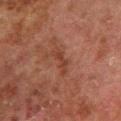{"biopsy_status": "not biopsied; imaged during a skin examination"}